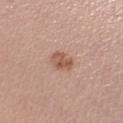Q: Was this lesion biopsied?
A: catalogued during a skin exam; not biopsied
Q: Where on the body is the lesion?
A: the left lower leg
Q: What did automated image analysis measure?
A: a lesion color around L≈55 a*≈21 b*≈29 in CIELAB, about 11 CIELAB-L* units darker than the surrounding skin, and a lesion-to-skin contrast of about 7.5 (normalized; higher = more distinct); a border-irregularity index near 2.5/10 and a color-variation rating of about 3.5/10; a nevus-likeness score of about 30/100 and a lesion-detection confidence of about 100/100
Q: How large is the lesion?
A: ~3 mm (longest diameter)
Q: Patient demographics?
A: female, in their mid-30s
Q: How was this image acquired?
A: total-body-photography crop, ~15 mm field of view